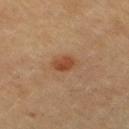Impression:
The lesion was tiled from a total-body skin photograph and was not biopsied.
Acquisition and patient details:
The recorded lesion diameter is about 2.5 mm. The lesion is located on the chest. A roughly 15 mm field-of-view crop from a total-body skin photograph. The patient is a female about 60 years old.